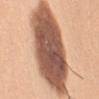• notes · total-body-photography surveillance lesion; no biopsy
• anatomic site · the arm
• imaging modality · 15 mm crop, total-body photography
• image-analysis metrics · a lesion area of about 55 mm², a shape eccentricity near 0.85, and a shape-asymmetry score of about 0.1 (0 = symmetric); a lesion color around L≈55 a*≈21 b*≈30 in CIELAB and a normalized lesion–skin contrast near 13; a border-irregularity index near 2/10, internal color variation of about 6.5 on a 0–10 scale, and radial color variation of about 2; a detector confidence of about 100 out of 100 that the crop contains a lesion
• size · ≈12 mm
• subject · female, about 45 years old
• illumination · white-light illumination